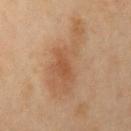Q: Is there a histopathology result?
A: catalogued during a skin exam; not biopsied
Q: What is the anatomic site?
A: the right upper arm
Q: What did automated image analysis measure?
A: a border-irregularity index near 6/10, a color-variation rating of about 4/10, and a peripheral color-asymmetry measure near 1
Q: Illumination type?
A: cross-polarized illumination
Q: How was this image acquired?
A: ~15 mm tile from a whole-body skin photo
Q: Patient demographics?
A: female, aged 43 to 47
Q: Lesion size?
A: ~9 mm (longest diameter)